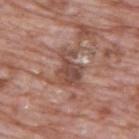Impression: The lesion was tiled from a total-body skin photograph and was not biopsied. Background: Located on the upper back. A male patient aged 68–72. This is a white-light tile. Cropped from a whole-body photographic skin survey; the tile spans about 15 mm. The recorded lesion diameter is about 4 mm. Automated image analysis of the tile measured a shape eccentricity near 0.8 and a shape-asymmetry score of about 0.4 (0 = symmetric).